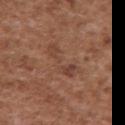The lesion was photographed on a routine skin check and not biopsied; there is no pathology result.
Cropped from a whole-body photographic skin survey; the tile spans about 15 mm.
An algorithmic analysis of the crop reported a footprint of about 8.5 mm² and a symmetry-axis asymmetry near 0.55. The analysis additionally found an average lesion color of about L≈44 a*≈21 b*≈28 (CIELAB), roughly 5 lightness units darker than nearby skin, and a normalized lesion–skin contrast near 4.5. And it measured peripheral color asymmetry of about 0.5. The software also gave a nevus-likeness score of about 0/100 and a detector confidence of about 100 out of 100 that the crop contains a lesion.
The subject is a male aged approximately 45.
From the upper back.
Captured under white-light illumination.
The lesion's longest dimension is about 5.5 mm.